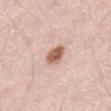{"biopsy_status": "not biopsied; imaged during a skin examination", "lesion_size": {"long_diameter_mm_approx": 3.0}, "lighting": "white-light", "patient": {"sex": "male", "age_approx": 70}, "automated_metrics": {"border_irregularity_0_10": 2.0, "color_variation_0_10": 3.5}, "site": "front of the torso", "image": {"source": "total-body photography crop", "field_of_view_mm": 15}}